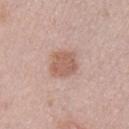No biopsy was performed on this lesion — it was imaged during a full skin examination and was not determined to be concerning.
An algorithmic analysis of the crop reported a lesion area of about 9 mm² and a shape eccentricity near 0.45.
Longest diameter approximately 3.5 mm.
A 15 mm close-up extracted from a 3D total-body photography capture.
The patient is a male approximately 30 years of age.